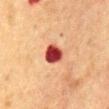follow-up — catalogued during a skin exam; not biopsied
lesion diameter — ≈2.5 mm
patient — female, aged 48 to 52
imaging modality — ~15 mm tile from a whole-body skin photo
anatomic site — the chest